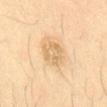The lesion was photographed on a routine skin check and not biopsied; there is no pathology result. Imaged with cross-polarized lighting. A 15 mm close-up tile from a total-body photography series done for melanoma screening. A male subject, about 35 years old. From the mid back.